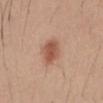{
  "biopsy_status": "not biopsied; imaged during a skin examination",
  "site": "abdomen",
  "image": {
    "source": "total-body photography crop",
    "field_of_view_mm": 15
  },
  "patient": {
    "sex": "male",
    "age_approx": 30
  }
}